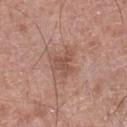Background: This image is a 15 mm lesion crop taken from a total-body photograph. The recorded lesion diameter is about 3 mm. A male subject aged 58 to 62. Automated tile analysis of the lesion measured an area of roughly 4.5 mm², an eccentricity of roughly 0.55, and a shape-asymmetry score of about 0.5 (0 = symmetric). And it measured a within-lesion color-variation index near 1/10 and a peripheral color-asymmetry measure near 0.5. It also reported a classifier nevus-likeness of about 0/100 and a detector confidence of about 100 out of 100 that the crop contains a lesion. Located on the left lower leg. Captured under white-light illumination.